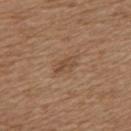Clinical impression:
The lesion was photographed on a routine skin check and not biopsied; there is no pathology result.
Background:
Cropped from a whole-body photographic skin survey; the tile spans about 15 mm. A female subject in their mid- to late 50s. From the upper back.